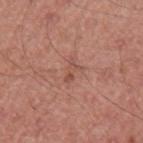A lesion tile, about 15 mm wide, cut from a 3D total-body photograph. The subject is a male aged 43–47. On the right upper arm.